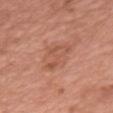Notes:
• lighting — white-light illumination
• patient — female, aged around 40
• size — about 4.5 mm
• automated lesion analysis — a normalized border contrast of about 5; an automated nevus-likeness rating near 5 out of 100 and a detector confidence of about 100 out of 100 that the crop contains a lesion
• body site — the front of the torso
• acquisition — ~15 mm crop, total-body skin-cancer survey